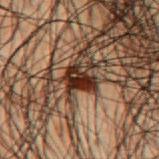The lesion was photographed on a routine skin check and not biopsied; there is no pathology result. Cropped from a total-body skin-imaging series; the visible field is about 15 mm. The total-body-photography lesion software estimated a shape eccentricity near 0.7. The software also gave a border-irregularity index near 3/10 and peripheral color asymmetry of about 2. A male subject, in their 50s. From the mid back.